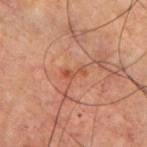Findings:
* site · the chest
* tile lighting · cross-polarized
* patient · male, aged around 70
* image source · ~15 mm tile from a whole-body skin photo